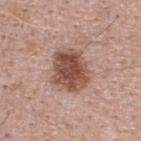Clinical impression:
This lesion was catalogued during total-body skin photography and was not selected for biopsy.
Clinical summary:
Approximately 4.5 mm at its widest. A male patient in their 40s. Captured under white-light illumination. A 15 mm close-up tile from a total-body photography series done for melanoma screening. Located on the back.